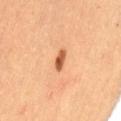workup: total-body-photography surveillance lesion; no biopsy
imaging modality: 15 mm crop, total-body photography
patient: female, in their 60s
TBP lesion metrics: a footprint of about 3 mm², an outline eccentricity of about 0.85 (0 = round, 1 = elongated), and a shape-asymmetry score of about 0.3 (0 = symmetric)
lesion diameter: ≈2.5 mm
site: the lower back
tile lighting: cross-polarized illumination